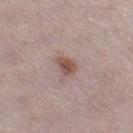Case summary:
* biopsy status — catalogued during a skin exam; not biopsied
* image source — 15 mm crop, total-body photography
* location — the leg
* illumination — white-light
* lesion diameter — ~2.5 mm (longest diameter)
* patient — female, approximately 30 years of age